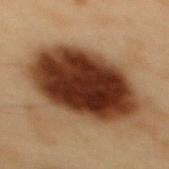Acquisition and patient details: An algorithmic analysis of the crop reported a footprint of about 50 mm² and a shape eccentricity near 0.7. The analysis additionally found a lesion color around L≈27 a*≈18 b*≈25 in CIELAB, about 19 CIELAB-L* units darker than the surrounding skin, and a lesion-to-skin contrast of about 17 (normalized; higher = more distinct). The lesion is located on the mid back. A 15 mm close-up tile from a total-body photography series done for melanoma screening. Captured under cross-polarized illumination. About 9.5 mm across. The patient is a male in their mid-50s.